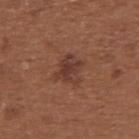Notes:
* workup — no biopsy performed (imaged during a skin exam)
* automated metrics — a classifier nevus-likeness of about 25/100 and lesion-presence confidence of about 100/100
* size — about 3 mm
* illumination — white-light illumination
* body site — the upper back
* image — ~15 mm crop, total-body skin-cancer survey
* subject — female, in their mid- to late 60s